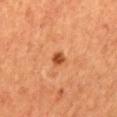Assessment: No biopsy was performed on this lesion — it was imaged during a full skin examination and was not determined to be concerning. Clinical summary: A male patient, about 65 years old. The tile uses cross-polarized illumination. Longest diameter approximately 2 mm. Automated tile analysis of the lesion measured a lesion color around L≈46 a*≈30 b*≈39 in CIELAB and a normalized lesion–skin contrast near 9.5. It also reported border irregularity of about 1.5 on a 0–10 scale, a color-variation rating of about 3/10, and radial color variation of about 1. The lesion is on the abdomen. A 15 mm crop from a total-body photograph taken for skin-cancer surveillance.